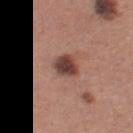Impression:
Part of a total-body skin-imaging series; this lesion was reviewed on a skin check and was not flagged for biopsy.
Acquisition and patient details:
A close-up tile cropped from a whole-body skin photograph, about 15 mm across. The subject is a female about 30 years old. Located on the left thigh.